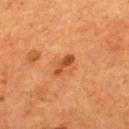follow-up: total-body-photography surveillance lesion; no biopsy | anatomic site: the upper back | subject: male, aged around 55 | acquisition: 15 mm crop, total-body photography.